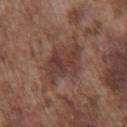Clinical impression:
Imaged during a routine full-body skin examination; the lesion was not biopsied and no histopathology is available.
Context:
The lesion is located on the chest. A male subject, in their mid- to late 70s. A close-up tile cropped from a whole-body skin photograph, about 15 mm across.